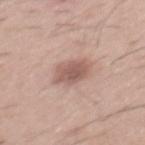Context:
A 15 mm crop from a total-body photograph taken for skin-cancer surveillance. The lesion is located on the mid back. Automated tile analysis of the lesion measured a lesion color around L≈57 a*≈20 b*≈24 in CIELAB, roughly 11 lightness units darker than nearby skin, and a normalized lesion–skin contrast near 7.5. The analysis additionally found a border-irregularity rating of about 1.5/10 and internal color variation of about 2.5 on a 0–10 scale. A male subject aged 28–32.